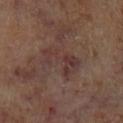No biopsy was performed on this lesion — it was imaged during a full skin examination and was not determined to be concerning. A region of skin cropped from a whole-body photographic capture, roughly 15 mm wide. The lesion's longest dimension is about 5 mm. Automated image analysis of the tile measured a footprint of about 12 mm², a shape eccentricity near 0.75, and a shape-asymmetry score of about 0.35 (0 = symmetric). The analysis additionally found an average lesion color of about L≈34 a*≈17 b*≈19 (CIELAB) and a normalized lesion–skin contrast near 6. The analysis additionally found internal color variation of about 5 on a 0–10 scale and a peripheral color-asymmetry measure near 2. It also reported an automated nevus-likeness rating near 0 out of 100 and a lesion-detection confidence of about 100/100. The subject is a male about 70 years old. Located on the left lower leg. Captured under cross-polarized illumination.